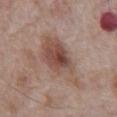workup = imaged on a skin check; not biopsied | acquisition = ~15 mm tile from a whole-body skin photo | lighting = white-light illumination | lesion size = ≈6.5 mm | anatomic site = the abdomen | patient = male, aged around 70.